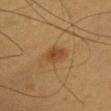• follow-up · imaged on a skin check; not biopsied
• automated lesion analysis · a mean CIELAB color near L≈41 a*≈20 b*≈33, roughly 8 lightness units darker than nearby skin, and a normalized lesion–skin contrast near 6.5; a nevus-likeness score of about 90/100 and a detector confidence of about 100 out of 100 that the crop contains a lesion
• body site · the chest
• tile lighting · cross-polarized
• lesion diameter · ≈3 mm
• patient · female, aged 28 to 32
• acquisition · total-body-photography crop, ~15 mm field of view